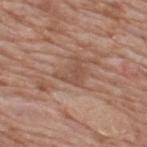{
  "biopsy_status": "not biopsied; imaged during a skin examination",
  "image": {
    "source": "total-body photography crop",
    "field_of_view_mm": 15
  },
  "lighting": "white-light",
  "lesion_size": {
    "long_diameter_mm_approx": 3.5
  },
  "patient": {
    "sex": "male",
    "age_approx": 60
  },
  "site": "upper back"
}